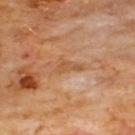Recorded during total-body skin imaging; not selected for excision or biopsy. The patient is a male about 60 years old. This image is a 15 mm lesion crop taken from a total-body photograph. On the front of the torso.